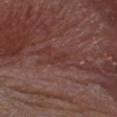The lesion is on the head or neck. A close-up tile cropped from a whole-body skin photograph, about 15 mm across. Longest diameter approximately 2.5 mm. Automated image analysis of the tile measured a lesion area of about 3 mm², a shape eccentricity near 0.9, and a symmetry-axis asymmetry near 0.35. The software also gave a lesion color around L≈35 a*≈23 b*≈22 in CIELAB and roughly 5 lightness units darker than nearby skin. It also reported an automated nevus-likeness rating near 0 out of 100 and a lesion-detection confidence of about 80/100. A male patient about 80 years old. This is a white-light tile.